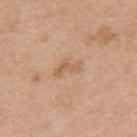Impression:
Part of a total-body skin-imaging series; this lesion was reviewed on a skin check and was not flagged for biopsy.
Clinical summary:
This is a white-light tile. This image is a 15 mm lesion crop taken from a total-body photograph. A female subject, in their 40s. The lesion is on the upper back. Approximately 3.5 mm at its widest.